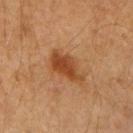Q: Was this lesion biopsied?
A: total-body-photography surveillance lesion; no biopsy
Q: Who is the patient?
A: male, aged approximately 60
Q: What kind of image is this?
A: 15 mm crop, total-body photography
Q: What is the anatomic site?
A: the right upper arm
Q: What is the lesion's diameter?
A: ~4.5 mm (longest diameter)